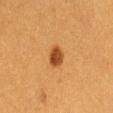No biopsy was performed on this lesion — it was imaged during a full skin examination and was not determined to be concerning.